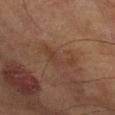Clinical impression:
The lesion was photographed on a routine skin check and not biopsied; there is no pathology result.
Context:
A 15 mm crop from a total-body photograph taken for skin-cancer surveillance. From the right lower leg. A male patient, in their mid- to late 80s or older.